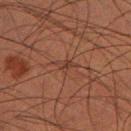Assessment:
Captured during whole-body skin photography for melanoma surveillance; the lesion was not biopsied.
Background:
The lesion is on the leg. The patient is a male aged 48 to 52. A 15 mm close-up tile from a total-body photography series done for melanoma screening. Measured at roughly 3 mm in maximum diameter.